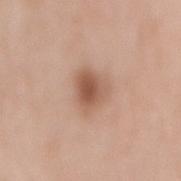workup = total-body-photography surveillance lesion; no biopsy
image = ~15 mm tile from a whole-body skin photo
location = the back
subject = female, in their 70s
lighting = white-light illumination
automated lesion analysis = a border-irregularity rating of about 2.5/10, a within-lesion color-variation index near 5.5/10, and radial color variation of about 1.5; a classifier nevus-likeness of about 95/100 and lesion-presence confidence of about 100/100